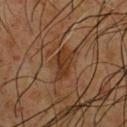Q: Patient demographics?
A: male, approximately 65 years of age
Q: What kind of image is this?
A: total-body-photography crop, ~15 mm field of view
Q: Lesion location?
A: the chest